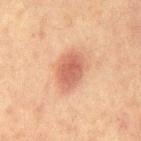  biopsy_status: not biopsied; imaged during a skin examination
  site: chest
  image:
    source: total-body photography crop
    field_of_view_mm: 15
  lesion_size:
    long_diameter_mm_approx: 5.0
  automated_metrics:
    border_irregularity_0_10: 1.5
    color_variation_0_10: 3.0
    lesion_detection_confidence_0_100: 100
  lighting: cross-polarized
  patient:
    sex: male
    age_approx: 65